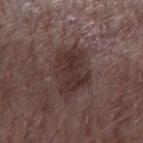Q: Was this lesion biopsied?
A: total-body-photography surveillance lesion; no biopsy
Q: Lesion size?
A: ~5.5 mm (longest diameter)
Q: How was this image acquired?
A: total-body-photography crop, ~15 mm field of view
Q: Lesion location?
A: the left forearm
Q: Automated lesion metrics?
A: a lesion area of about 17 mm², a shape eccentricity near 0.75, and a symmetry-axis asymmetry near 0.2; a classifier nevus-likeness of about 40/100 and a lesion-detection confidence of about 100/100
Q: What are the patient's age and sex?
A: male, aged 63 to 67
Q: Illumination type?
A: white-light illumination